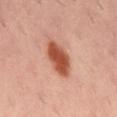Case summary:
- workup: imaged on a skin check; not biopsied
- anatomic site: the back
- imaging modality: ~15 mm crop, total-body skin-cancer survey
- lesion diameter: ≈5 mm
- patient: male, aged around 40
- automated lesion analysis: a lesion color around L≈51 a*≈28 b*≈33 in CIELAB, a lesion–skin lightness drop of about 16, and a normalized lesion–skin contrast near 11; a border-irregularity rating of about 1.5/10, a color-variation rating of about 3/10, and a peripheral color-asymmetry measure near 1